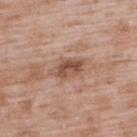Context:
An algorithmic analysis of the crop reported a lesion area of about 7 mm². It also reported a mean CIELAB color near L≈51 a*≈20 b*≈28, a lesion–skin lightness drop of about 12, and a normalized border contrast of about 8. It also reported a classifier nevus-likeness of about 5/100. Located on the upper back. This is a white-light tile. Measured at roughly 4 mm in maximum diameter. A male subject, approximately 50 years of age. Cropped from a whole-body photographic skin survey; the tile spans about 15 mm.